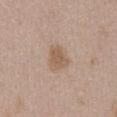Captured under white-light illumination. A male patient in their 50s. Located on the front of the torso. A roughly 15 mm field-of-view crop from a total-body skin photograph. The recorded lesion diameter is about 3 mm.